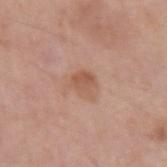Assessment: Captured during whole-body skin photography for melanoma surveillance; the lesion was not biopsied. Background: A male subject approximately 60 years of age. From the left upper arm. A lesion tile, about 15 mm wide, cut from a 3D total-body photograph. The tile uses white-light illumination.